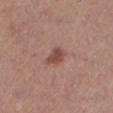Notes:
- biopsy status — total-body-photography surveillance lesion; no biopsy
- subject — female, roughly 65 years of age
- diameter — about 2.5 mm
- lighting — white-light illumination
- anatomic site — the left lower leg
- acquisition — ~15 mm crop, total-body skin-cancer survey